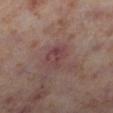Assessment: No biopsy was performed on this lesion — it was imaged during a full skin examination and was not determined to be concerning. Image and clinical context: Imaged with cross-polarized lighting. A region of skin cropped from a whole-body photographic capture, roughly 15 mm wide. Longest diameter approximately 3.5 mm. The subject is a female in their 40s. From the leg.